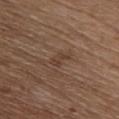Part of a total-body skin-imaging series; this lesion was reviewed on a skin check and was not flagged for biopsy.
This image is a 15 mm lesion crop taken from a total-body photograph.
The lesion is located on the chest.
A male patient, aged 63 to 67.